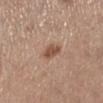follow-up = catalogued during a skin exam; not biopsied | anatomic site = the left lower leg | lesion diameter = about 3 mm | acquisition = ~15 mm tile from a whole-body skin photo | subject = female, aged 63–67 | tile lighting = white-light.